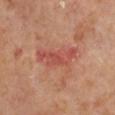Imaged during a routine full-body skin examination; the lesion was not biopsied and no histopathology is available. Cropped from a total-body skin-imaging series; the visible field is about 15 mm. The total-body-photography lesion software estimated an automated nevus-likeness rating near 0 out of 100. A female patient, roughly 60 years of age. This is a cross-polarized tile. The lesion is on the chest.